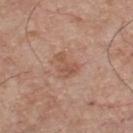follow-up: imaged on a skin check; not biopsied | tile lighting: white-light | subject: male, aged around 75 | site: the front of the torso | lesion size: ~3 mm (longest diameter) | acquisition: ~15 mm crop, total-body skin-cancer survey.